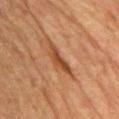Q: Is there a histopathology result?
A: imaged on a skin check; not biopsied
Q: What are the patient's age and sex?
A: male, aged 78 to 82
Q: What is the imaging modality?
A: ~15 mm tile from a whole-body skin photo
Q: What did automated image analysis measure?
A: an area of roughly 5 mm² and two-axis asymmetry of about 0.45; about 9 CIELAB-L* units darker than the surrounding skin
Q: Lesion location?
A: the mid back
Q: How large is the lesion?
A: ~4.5 mm (longest diameter)